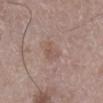This lesion was catalogued during total-body skin photography and was not selected for biopsy. A lesion tile, about 15 mm wide, cut from a 3D total-body photograph. The lesion is located on the right lower leg. The total-body-photography lesion software estimated a footprint of about 5.5 mm², an outline eccentricity of about 0.65 (0 = round, 1 = elongated), and two-axis asymmetry of about 0.25. And it measured a border-irregularity index near 2.5/10, internal color variation of about 2.5 on a 0–10 scale, and radial color variation of about 1. The software also gave lesion-presence confidence of about 100/100. This is a white-light tile. The patient is a male roughly 50 years of age.